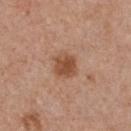  biopsy_status: not biopsied; imaged during a skin examination
  image:
    source: total-body photography crop
    field_of_view_mm: 15
  patient:
    sex: female
    age_approx: 35
  lighting: white-light
  site: front of the torso
  automated_metrics:
    area_mm2_approx: 7.0
    eccentricity: 0.4
    shape_asymmetry: 0.15
    nevus_likeness_0_100: 85
    lesion_detection_confidence_0_100: 100
  lesion_size:
    long_diameter_mm_approx: 3.0